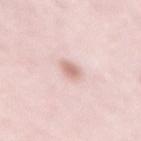Recorded during total-body skin imaging; not selected for excision or biopsy.
The lesion is located on the mid back.
This image is a 15 mm lesion crop taken from a total-body photograph.
The tile uses white-light illumination.
Automated tile analysis of the lesion measured an automated nevus-likeness rating near 90 out of 100 and lesion-presence confidence of about 100/100.
The subject is a female roughly 40 years of age.